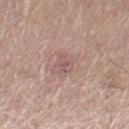Case summary:
* follow-up: no biopsy performed (imaged during a skin exam)
* image: ~15 mm crop, total-body skin-cancer survey
* diameter: ~2.5 mm (longest diameter)
* site: the left thigh
* lighting: white-light illumination
* subject: male, in their mid- to late 60s